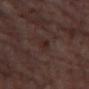Q: What kind of image is this?
A: ~15 mm crop, total-body skin-cancer survey
Q: What did automated image analysis measure?
A: an area of roughly 2.5 mm², a shape eccentricity near 0.95, and a shape-asymmetry score of about 0.25 (0 = symmetric); a mean CIELAB color near L≈25 a*≈17 b*≈19 and about 5 CIELAB-L* units darker than the surrounding skin
Q: How was the tile lit?
A: cross-polarized
Q: Where on the body is the lesion?
A: the left thigh
Q: What are the patient's age and sex?
A: female, aged approximately 55
Q: How large is the lesion?
A: about 3 mm